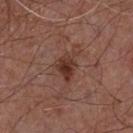A 15 mm crop from a total-body photograph taken for skin-cancer surveillance.
A male subject, aged 53 to 57.
Measured at roughly 3.5 mm in maximum diameter.
Located on the chest.
The tile uses white-light illumination.